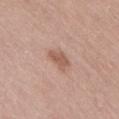workup=imaged on a skin check; not biopsied | patient=female, aged around 40 | site=the right thigh | imaging modality=total-body-photography crop, ~15 mm field of view | illumination=white-light | image-analysis metrics=a classifier nevus-likeness of about 50/100 and a lesion-detection confidence of about 100/100.